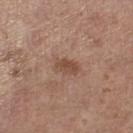Case summary:
– follow-up — catalogued during a skin exam; not biopsied
– tile lighting — white-light
– lesion diameter — ~3 mm (longest diameter)
– TBP lesion metrics — an average lesion color of about L≈48 a*≈20 b*≈28 (CIELAB), roughly 9 lightness units darker than nearby skin, and a normalized border contrast of about 7; a border-irregularity rating of about 1.5/10, a color-variation rating of about 2/10, and a peripheral color-asymmetry measure near 1
– body site — the leg
– image — ~15 mm crop, total-body skin-cancer survey
– subject — female, aged 63–67